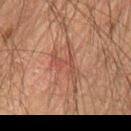{
  "biopsy_status": "not biopsied; imaged during a skin examination",
  "image": {
    "source": "total-body photography crop",
    "field_of_view_mm": 15
  },
  "lesion_size": {
    "long_diameter_mm_approx": 4.5
  },
  "lighting": "cross-polarized",
  "patient": {
    "sex": "male",
    "age_approx": 65
  },
  "site": "lower back"
}